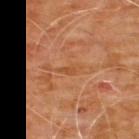Impression:
Captured during whole-body skin photography for melanoma surveillance; the lesion was not biopsied.
Image and clinical context:
An algorithmic analysis of the crop reported an average lesion color of about L≈49 a*≈24 b*≈38 (CIELAB) and a lesion-to-skin contrast of about 5.5 (normalized; higher = more distinct). The software also gave a border-irregularity rating of about 4/10, a within-lesion color-variation index near 0/10, and radial color variation of about 0. A close-up tile cropped from a whole-body skin photograph, about 15 mm across. About 2.5 mm across. Captured under cross-polarized illumination. The lesion is located on the chest. A male patient, aged 58–62.